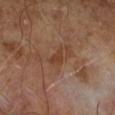This is a cross-polarized tile. Cropped from a total-body skin-imaging series; the visible field is about 15 mm. A male patient, about 65 years old.